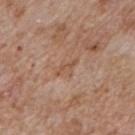| feature | finding |
|---|---|
| biopsy status | total-body-photography surveillance lesion; no biopsy |
| TBP lesion metrics | roughly 6 lightness units darker than nearby skin and a lesion-to-skin contrast of about 5.5 (normalized; higher = more distinct); a border-irregularity index near 4.5/10, internal color variation of about 0 on a 0–10 scale, and peripheral color asymmetry of about 0; an automated nevus-likeness rating near 0 out of 100 and a lesion-detection confidence of about 95/100 |
| location | the mid back |
| illumination | white-light illumination |
| imaging modality | ~15 mm tile from a whole-body skin photo |
| subject | male, approximately 60 years of age |